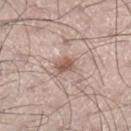<tbp_lesion>
  <biopsy_status>not biopsied; imaged during a skin examination</biopsy_status>
  <patient>
    <sex>male</sex>
    <age_approx>30</age_approx>
  </patient>
  <image>
    <source>total-body photography crop</source>
    <field_of_view_mm>15</field_of_view_mm>
  </image>
  <automated_metrics>
    <peripheral_color_asymmetry>1.0</peripheral_color_asymmetry>
    <nevus_likeness_0_100>55</nevus_likeness_0_100>
    <lesion_detection_confidence_0_100>100</lesion_detection_confidence_0_100>
  </automated_metrics>
  <lesion_size>
    <long_diameter_mm_approx>2.5</long_diameter_mm_approx>
  </lesion_size>
  <site>right lower leg</site>
  <lighting>white-light</lighting>
</tbp_lesion>